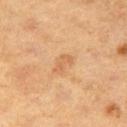biopsy status = total-body-photography surveillance lesion; no biopsy
location = the leg
imaging modality = total-body-photography crop, ~15 mm field of view
subject = female, aged approximately 40